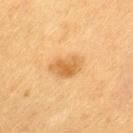Notes:
• follow-up: total-body-photography surveillance lesion; no biopsy
• location: the left thigh
• TBP lesion metrics: a lesion area of about 8 mm², a shape eccentricity near 0.65, and two-axis asymmetry of about 0.25; a mean CIELAB color near L≈54 a*≈19 b*≈40 and a normalized lesion–skin contrast near 6.5
• image: ~15 mm crop, total-body skin-cancer survey
• subject: female, aged around 55
• tile lighting: cross-polarized
• lesion diameter: about 4 mm